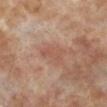Findings:
* notes · imaged on a skin check; not biopsied
* automated lesion analysis · a lesion area of about 5.5 mm², a shape eccentricity near 0.75, and a symmetry-axis asymmetry near 0.5
* lighting · cross-polarized
* body site · the left lower leg
* lesion size · about 3.5 mm
* acquisition · ~15 mm tile from a whole-body skin photo
* patient · male, roughly 70 years of age